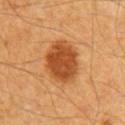Q: Is there a histopathology result?
A: total-body-photography surveillance lesion; no biopsy
Q: What did automated image analysis measure?
A: a mean CIELAB color near L≈45 a*≈26 b*≈40 and a normalized border contrast of about 10
Q: Where on the body is the lesion?
A: the chest
Q: How large is the lesion?
A: about 5 mm
Q: What lighting was used for the tile?
A: cross-polarized illumination
Q: What is the imaging modality?
A: total-body-photography crop, ~15 mm field of view
Q: What are the patient's age and sex?
A: male, aged around 60